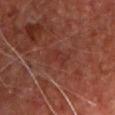<lesion>
  <biopsy_status>not biopsied; imaged during a skin examination</biopsy_status>
</lesion>